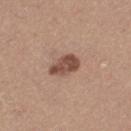Recorded during total-body skin imaging; not selected for excision or biopsy.
The recorded lesion diameter is about 4 mm.
This is a white-light tile.
The lesion is on the leg.
A lesion tile, about 15 mm wide, cut from a 3D total-body photograph.
A female subject aged 43–47.